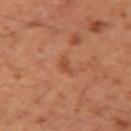The lesion-visualizer software estimated a footprint of about 2.5 mm², a shape eccentricity near 0.9, and two-axis asymmetry of about 0.4.
A close-up tile cropped from a whole-body skin photograph, about 15 mm across.
On the left upper arm.
This is a cross-polarized tile.
A male subject approximately 50 years of age.